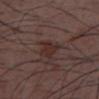Q: Is there a histopathology result?
A: imaged on a skin check; not biopsied
Q: How was this image acquired?
A: total-body-photography crop, ~15 mm field of view
Q: Patient demographics?
A: male, aged approximately 30
Q: What did automated image analysis measure?
A: a lesion color around L≈29 a*≈17 b*≈19 in CIELAB, roughly 6 lightness units darker than nearby skin, and a normalized lesion–skin contrast near 7; a classifier nevus-likeness of about 25/100 and a lesion-detection confidence of about 100/100
Q: Where on the body is the lesion?
A: the chest
Q: Illumination type?
A: white-light illumination
Q: How large is the lesion?
A: ~3 mm (longest diameter)